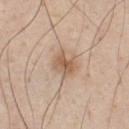site: the chest; lesion size: ≈3 mm; subject: male, in their mid- to late 40s; acquisition: ~15 mm crop, total-body skin-cancer survey; tile lighting: white-light.